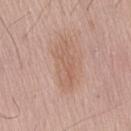This lesion was catalogued during total-body skin photography and was not selected for biopsy. The recorded lesion diameter is about 5.5 mm. The patient is a male aged 63 to 67. A roughly 15 mm field-of-view crop from a total-body skin photograph. Located on the lower back.